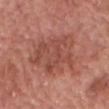This lesion was catalogued during total-body skin photography and was not selected for biopsy. Imaged with white-light lighting. A male subject, aged 78 to 82. Located on the chest. A 15 mm crop from a total-body photograph taken for skin-cancer surveillance. The total-body-photography lesion software estimated a footprint of about 27 mm², an eccentricity of roughly 0.5, and a symmetry-axis asymmetry near 0.3. And it measured border irregularity of about 4.5 on a 0–10 scale, a within-lesion color-variation index near 4/10, and a peripheral color-asymmetry measure near 1.5. The software also gave an automated nevus-likeness rating near 0 out of 100 and a detector confidence of about 100 out of 100 that the crop contains a lesion.